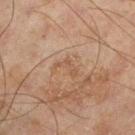A roughly 15 mm field-of-view crop from a total-body skin photograph.
The lesion is located on the left lower leg.
An algorithmic analysis of the crop reported a mean CIELAB color near L≈43 a*≈16 b*≈27 and a lesion-to-skin contrast of about 4.5 (normalized; higher = more distinct).
The subject is a male in their mid-40s.